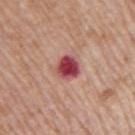workup = total-body-photography surveillance lesion; no biopsy
acquisition = ~15 mm tile from a whole-body skin photo
subject = male, roughly 70 years of age
lighting = white-light illumination
body site = the right upper arm